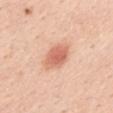workup — total-body-photography surveillance lesion; no biopsy | automated lesion analysis — a lesion area of about 7.5 mm², an eccentricity of roughly 0.75, and a symmetry-axis asymmetry near 0.2; an average lesion color of about L≈65 a*≈27 b*≈33 (CIELAB), about 12 CIELAB-L* units darker than the surrounding skin, and a lesion-to-skin contrast of about 7.5 (normalized; higher = more distinct); an automated nevus-likeness rating near 100 out of 100 | anatomic site — the back | size — about 4 mm | tile lighting — white-light | image — total-body-photography crop, ~15 mm field of view | subject — female, aged approximately 45.